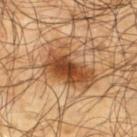biopsy status: imaged on a skin check; not biopsied | patient: male, in their mid-60s | image-analysis metrics: a footprint of about 18 mm², an outline eccentricity of about 0.8 (0 = round, 1 = elongated), and a symmetry-axis asymmetry near 0.35; about 15 CIELAB-L* units darker than the surrounding skin and a normalized lesion–skin contrast near 10.5; border irregularity of about 4.5 on a 0–10 scale and peripheral color asymmetry of about 1.5; a nevus-likeness score of about 90/100 and a detector confidence of about 100 out of 100 that the crop contains a lesion | lighting: cross-polarized | location: the upper back | acquisition: ~15 mm crop, total-body skin-cancer survey.